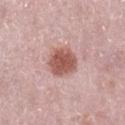Impression: No biopsy was performed on this lesion — it was imaged during a full skin examination and was not determined to be concerning. Acquisition and patient details: A female patient roughly 40 years of age. The lesion is located on the right lower leg. A lesion tile, about 15 mm wide, cut from a 3D total-body photograph.